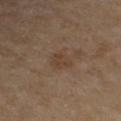On the arm. The total-body-photography lesion software estimated a lesion area of about 4 mm², an outline eccentricity of about 0.7 (0 = round, 1 = elongated), and a symmetry-axis asymmetry near 0.4. And it measured a border-irregularity index near 4/10 and internal color variation of about 1 on a 0–10 scale. Captured under cross-polarized illumination. Cropped from a total-body skin-imaging series; the visible field is about 15 mm. The patient is a female aged 58–62.